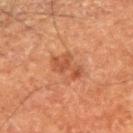Clinical impression: The lesion was tiled from a total-body skin photograph and was not biopsied. Context: Located on the right upper arm. A lesion tile, about 15 mm wide, cut from a 3D total-body photograph. Captured under cross-polarized illumination. A male subject aged 73–77.